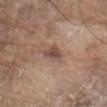Cropped from a whole-body photographic skin survey; the tile spans about 15 mm.
A male subject aged approximately 80.
The lesion is on the abdomen.